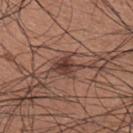Q: Was a biopsy performed?
A: catalogued during a skin exam; not biopsied
Q: Where on the body is the lesion?
A: the right upper arm
Q: How large is the lesion?
A: ~3.5 mm (longest diameter)
Q: How was the tile lit?
A: white-light
Q: What are the patient's age and sex?
A: male, aged around 35
Q: What is the imaging modality?
A: ~15 mm crop, total-body skin-cancer survey
Q: Automated lesion metrics?
A: an average lesion color of about L≈39 a*≈20 b*≈24 (CIELAB) and a lesion-to-skin contrast of about 10 (normalized; higher = more distinct); a border-irregularity rating of about 3.5/10, internal color variation of about 3.5 on a 0–10 scale, and peripheral color asymmetry of about 1.5